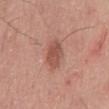No biopsy was performed on this lesion — it was imaged during a full skin examination and was not determined to be concerning.
A 15 mm close-up tile from a total-body photography series done for melanoma screening.
The recorded lesion diameter is about 3.5 mm.
An algorithmic analysis of the crop reported an outline eccentricity of about 0.7 (0 = round, 1 = elongated) and two-axis asymmetry of about 0.15.
A male patient aged 53 to 57.
The lesion is located on the mid back.
This is a white-light tile.